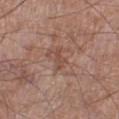acquisition=~15 mm crop, total-body skin-cancer survey
body site=the left lower leg
automated lesion analysis=a nevus-likeness score of about 0/100 and lesion-presence confidence of about 95/100
lesion size=≈3 mm
patient=male, aged approximately 60
lighting=white-light